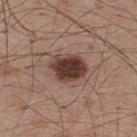Clinical impression:
The lesion was tiled from a total-body skin photograph and was not biopsied.
Background:
The patient is a male approximately 50 years of age. A lesion tile, about 15 mm wide, cut from a 3D total-body photograph. The lesion is on the upper back.